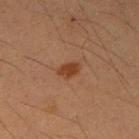follow-up — catalogued during a skin exam; not biopsied
tile lighting — cross-polarized
patient — male, roughly 35 years of age
size — ~3 mm (longest diameter)
imaging modality — 15 mm crop, total-body photography
anatomic site — the right upper arm